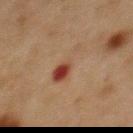Assessment:
Part of a total-body skin-imaging series; this lesion was reviewed on a skin check and was not flagged for biopsy.
Clinical summary:
Automated tile analysis of the lesion measured two-axis asymmetry of about 0.5. A male subject, aged 73 to 77. Measured at roughly 4.5 mm in maximum diameter. On the front of the torso. A 15 mm close-up tile from a total-body photography series done for melanoma screening. Imaged with cross-polarized lighting.